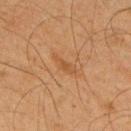Captured during whole-body skin photography for melanoma surveillance; the lesion was not biopsied. Cropped from a total-body skin-imaging series; the visible field is about 15 mm. The lesion is located on the back. Imaged with cross-polarized lighting. A male patient, in their mid- to late 60s. Automated tile analysis of the lesion measured a footprint of about 3 mm² and a symmetry-axis asymmetry near 0.4. The analysis additionally found a nevus-likeness score of about 0/100 and a lesion-detection confidence of about 100/100. The recorded lesion diameter is about 3 mm.